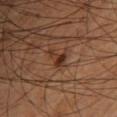workup: no biopsy performed (imaged during a skin exam)
site: the leg
subject: male, aged around 60
lighting: cross-polarized
image source: 15 mm crop, total-body photography
lesion size: ~3.5 mm (longest diameter)